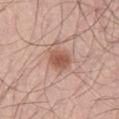A region of skin cropped from a whole-body photographic capture, roughly 15 mm wide. On the right thigh. A male patient, aged approximately 70. Captured under white-light illumination. The lesion's longest dimension is about 3.5 mm. The lesion-visualizer software estimated a lesion color around L≈56 a*≈22 b*≈28 in CIELAB, about 11 CIELAB-L* units darker than the surrounding skin, and a normalized border contrast of about 7.5. And it measured a classifier nevus-likeness of about 95/100.